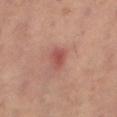<case>
  <biopsy_status>not biopsied; imaged during a skin examination</biopsy_status>
  <patient>
    <sex>female</sex>
    <age_approx>40</age_approx>
  </patient>
  <site>right thigh</site>
  <lesion_size>
    <long_diameter_mm_approx>3.0</long_diameter_mm_approx>
  </lesion_size>
  <image>
    <source>total-body photography crop</source>
    <field_of_view_mm>15</field_of_view_mm>
  </image>
  <automated_metrics>
    <area_mm2_approx>4.5</area_mm2_approx>
    <eccentricity>0.7</eccentricity>
    <shape_asymmetry>0.2</shape_asymmetry>
    <cielab_L>50</cielab_L>
    <cielab_a>27</cielab_a>
    <cielab_b>25</cielab_b>
    <vs_skin_darker_L>9.0</vs_skin_darker_L>
    <vs_skin_contrast_norm>6.5</vs_skin_contrast_norm>
    <border_irregularity_0_10>2.0</border_irregularity_0_10>
    <color_variation_0_10>2.0</color_variation_0_10>
    <peripheral_color_asymmetry>0.5</peripheral_color_asymmetry>
    <lesion_detection_confidence_0_100>100</lesion_detection_confidence_0_100>
  </automated_metrics>
</case>